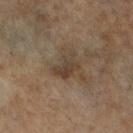  biopsy_status: not biopsied; imaged during a skin examination
  patient:
    sex: female
    age_approx: 65
  site: left leg
  lighting: cross-polarized
  image:
    source: total-body photography crop
    field_of_view_mm: 15
  lesion_size:
    long_diameter_mm_approx: 3.5